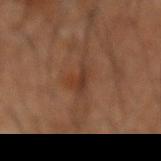No biopsy was performed on this lesion — it was imaged during a full skin examination and was not determined to be concerning.
From the mid back.
This image is a 15 mm lesion crop taken from a total-body photograph.
A male subject aged approximately 60.